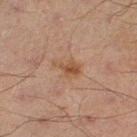biopsy_status: not biopsied; imaged during a skin examination
site: left thigh
patient:
  sex: male
  age_approx: 70
image:
  source: total-body photography crop
  field_of_view_mm: 15
lesion_size:
  long_diameter_mm_approx: 3.5
automated_metrics:
  area_mm2_approx: 5.5
  shape_asymmetry: 0.3
  vs_skin_contrast_norm: 6.5
  nevus_likeness_0_100: 10
lighting: cross-polarized